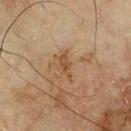No biopsy was performed on this lesion — it was imaged during a full skin examination and was not determined to be concerning. A 15 mm close-up extracted from a 3D total-body photography capture. A male patient aged 68–72. Imaged with cross-polarized lighting. The lesion-visualizer software estimated an area of roughly 3 mm² and two-axis asymmetry of about 0.3. The software also gave a mean CIELAB color near L≈40 a*≈16 b*≈28 and a lesion-to-skin contrast of about 6.5 (normalized; higher = more distinct). It also reported a detector confidence of about 100 out of 100 that the crop contains a lesion. The lesion is on the chest.